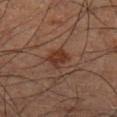The lesion was tiled from a total-body skin photograph and was not biopsied. Imaged with cross-polarized lighting. Located on the left lower leg. The lesion-visualizer software estimated lesion-presence confidence of about 100/100. The patient is a male roughly 60 years of age. Cropped from a whole-body photographic skin survey; the tile spans about 15 mm.